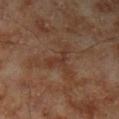Impression:
Imaged during a routine full-body skin examination; the lesion was not biopsied and no histopathology is available.
Context:
A roughly 15 mm field-of-view crop from a total-body skin photograph. On the leg. Approximately 4 mm at its widest. A male patient, aged around 70. An algorithmic analysis of the crop reported a lesion-detection confidence of about 100/100. This is a cross-polarized tile.